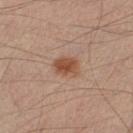Captured during whole-body skin photography for melanoma surveillance; the lesion was not biopsied. Automated tile analysis of the lesion measured an eccentricity of roughly 0.65 and a shape-asymmetry score of about 0.15 (0 = symmetric). And it measured a lesion-detection confidence of about 100/100. A male subject, aged approximately 35. The lesion is located on the leg. A close-up tile cropped from a whole-body skin photograph, about 15 mm across. The recorded lesion diameter is about 3 mm. Captured under cross-polarized illumination.